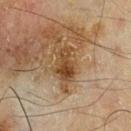  biopsy_status: not biopsied; imaged during a skin examination
  lesion_size:
    long_diameter_mm_approx: 4.0
  lighting: cross-polarized
  automated_metrics:
    area_mm2_approx: 6.0
    shape_asymmetry: 0.35
    cielab_L: 35
    cielab_a: 16
    cielab_b: 29
    vs_skin_darker_L: 10.0
    vs_skin_contrast_norm: 9.0
    nevus_likeness_0_100: 10
    lesion_detection_confidence_0_100: 100
  site: chest
  image:
    source: total-body photography crop
    field_of_view_mm: 15
  patient:
    sex: male
    age_approx: 70